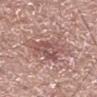Captured during whole-body skin photography for melanoma surveillance; the lesion was not biopsied.
The patient is a male aged 58–62.
An algorithmic analysis of the crop reported a footprint of about 20 mm² and a shape-asymmetry score of about 0.35 (0 = symmetric).
Cropped from a whole-body photographic skin survey; the tile spans about 15 mm.
Longest diameter approximately 7 mm.
This is a white-light tile.
The lesion is located on the left lower leg.